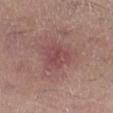Q: Is there a histopathology result?
A: catalogued during a skin exam; not biopsied
Q: How was this image acquired?
A: ~15 mm tile from a whole-body skin photo
Q: Lesion location?
A: the right lower leg
Q: Patient demographics?
A: male, about 55 years old
Q: What is the lesion's diameter?
A: ≈3.5 mm